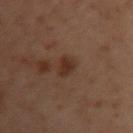No biopsy was performed on this lesion — it was imaged during a full skin examination and was not determined to be concerning. A female patient, aged approximately 55. Located on the left arm. A 15 mm crop from a total-body photograph taken for skin-cancer surveillance.